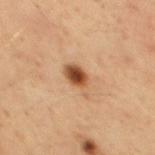follow-up=imaged on a skin check; not biopsied
subject=male, aged approximately 50
body site=the mid back
tile lighting=cross-polarized illumination
automated metrics=an automated nevus-likeness rating near 100 out of 100 and a lesion-detection confidence of about 100/100
diameter=~3.5 mm (longest diameter)
imaging modality=~15 mm tile from a whole-body skin photo